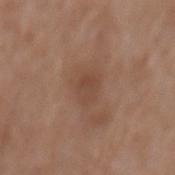Impression:
The lesion was tiled from a total-body skin photograph and was not biopsied.
Acquisition and patient details:
Measured at roughly 3 mm in maximum diameter. A roughly 15 mm field-of-view crop from a total-body skin photograph. On the mid back. A male subject aged around 75.